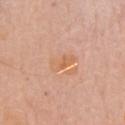{
  "biopsy_status": "not biopsied; imaged during a skin examination",
  "patient": {
    "sex": "male",
    "age_approx": 80
  },
  "lighting": "white-light",
  "lesion_size": {
    "long_diameter_mm_approx": 3.5
  },
  "site": "chest",
  "automated_metrics": {
    "color_variation_0_10": 3.0,
    "nevus_likeness_0_100": 0,
    "lesion_detection_confidence_0_100": 100
  },
  "image": {
    "source": "total-body photography crop",
    "field_of_view_mm": 15
  }
}